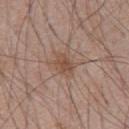follow-up = imaged on a skin check; not biopsied
image = ~15 mm tile from a whole-body skin photo
patient = male, approximately 70 years of age
location = the chest